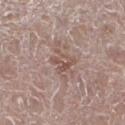Notes:
– notes · no biopsy performed (imaged during a skin exam)
– size · ~3 mm (longest diameter)
– image source · 15 mm crop, total-body photography
– patient · male, approximately 65 years of age
– site · the left lower leg
– tile lighting · white-light